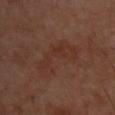Notes:
* subject · male, about 50 years old
* size · ~7 mm (longest diameter)
* illumination · cross-polarized illumination
* imaging modality · total-body-photography crop, ~15 mm field of view
* TBP lesion metrics · a shape eccentricity near 0.95 and a symmetry-axis asymmetry near 0.6; a lesion color around L≈31 a*≈20 b*≈24 in CIELAB; internal color variation of about 2.5 on a 0–10 scale and radial color variation of about 0.5
* body site · the upper back